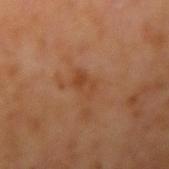Impression: Imaged during a routine full-body skin examination; the lesion was not biopsied and no histopathology is available. Clinical summary: Automated tile analysis of the lesion measured a mean CIELAB color near L≈41 a*≈22 b*≈33 and a lesion–skin lightness drop of about 6. It also reported a border-irregularity rating of about 3/10 and internal color variation of about 4.5 on a 0–10 scale. This is a cross-polarized tile. A 15 mm close-up tile from a total-body photography series done for melanoma screening. A male subject approximately 60 years of age. The lesion's longest dimension is about 3 mm. The lesion is on the right upper arm.